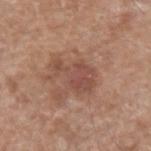Q: Patient demographics?
A: male, in their 60s
Q: How was this image acquired?
A: 15 mm crop, total-body photography
Q: What did automated image analysis measure?
A: a shape eccentricity near 0.8 and a symmetry-axis asymmetry near 0.45; an average lesion color of about L≈49 a*≈22 b*≈26 (CIELAB) and a lesion–skin lightness drop of about 8; a border-irregularity rating of about 6.5/10, a within-lesion color-variation index near 2.5/10, and radial color variation of about 0.5; a nevus-likeness score of about 10/100
Q: Lesion location?
A: the left forearm